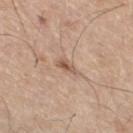A male patient, in their 70s. From the leg. Measured at roughly 3 mm in maximum diameter. An algorithmic analysis of the crop reported a lesion area of about 3.5 mm². The analysis additionally found a border-irregularity index near 3.5/10, internal color variation of about 3 on a 0–10 scale, and radial color variation of about 1. Imaged with white-light lighting. Cropped from a whole-body photographic skin survey; the tile spans about 15 mm.